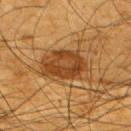Case summary:
- workup · total-body-photography surveillance lesion; no biopsy
- image-analysis metrics · an average lesion color of about L≈36 a*≈19 b*≈34 (CIELAB), roughly 10 lightness units darker than nearby skin, and a normalized lesion–skin contrast near 8.5; a border-irregularity rating of about 3/10, internal color variation of about 5 on a 0–10 scale, and radial color variation of about 1.5; an automated nevus-likeness rating near 80 out of 100 and a lesion-detection confidence of about 95/100
- body site · the right upper arm
- patient · male, about 65 years old
- illumination · cross-polarized illumination
- acquisition · ~15 mm tile from a whole-body skin photo
- lesion size · about 6.5 mm